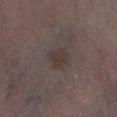Imaged during a routine full-body skin examination; the lesion was not biopsied and no histopathology is available. A 15 mm close-up tile from a total-body photography series done for melanoma screening. About 3 mm across. An algorithmic analysis of the crop reported a mean CIELAB color near L≈31 a*≈10 b*≈16, roughly 5 lightness units darker than nearby skin, and a normalized border contrast of about 6. It also reported border irregularity of about 1.5 on a 0–10 scale and a color-variation rating of about 2/10. The software also gave a classifier nevus-likeness of about 5/100 and lesion-presence confidence of about 100/100. From the right leg. The patient is a female about 80 years old.